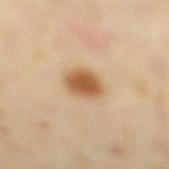Background: Imaged with cross-polarized lighting. The lesion is located on the leg. Cropped from a total-body skin-imaging series; the visible field is about 15 mm. A female patient, in their mid-30s. The recorded lesion diameter is about 3.5 mm.